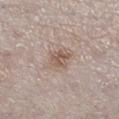Q: Is there a histopathology result?
A: catalogued during a skin exam; not biopsied
Q: What kind of image is this?
A: total-body-photography crop, ~15 mm field of view
Q: Lesion location?
A: the left lower leg
Q: What are the patient's age and sex?
A: female, in their 40s
Q: What lighting was used for the tile?
A: white-light
Q: Automated lesion metrics?
A: a footprint of about 4.5 mm² and an outline eccentricity of about 0.55 (0 = round, 1 = elongated); a border-irregularity rating of about 3/10, internal color variation of about 3 on a 0–10 scale, and a peripheral color-asymmetry measure near 1